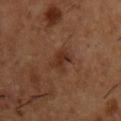Q: Is there a histopathology result?
A: catalogued during a skin exam; not biopsied
Q: What are the patient's age and sex?
A: male, aged around 55
Q: What is the imaging modality?
A: ~15 mm crop, total-body skin-cancer survey
Q: What lighting was used for the tile?
A: cross-polarized
Q: What is the lesion's diameter?
A: ≈3 mm
Q: Where on the body is the lesion?
A: the chest